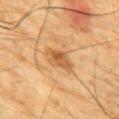No biopsy was performed on this lesion — it was imaged during a full skin examination and was not determined to be concerning. From the front of the torso. A 15 mm close-up extracted from a 3D total-body photography capture. Automated tile analysis of the lesion measured a within-lesion color-variation index near 4/10 and radial color variation of about 1.5. Imaged with cross-polarized lighting. The patient is a male about 85 years old or older. The lesion's longest dimension is about 3.5 mm.